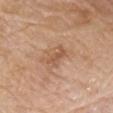| key | value |
|---|---|
| notes | no biopsy performed (imaged during a skin exam) |
| size | about 3.5 mm |
| patient | male, aged 78–82 |
| anatomic site | the chest |
| lighting | white-light |
| image source | ~15 mm crop, total-body skin-cancer survey |
| image-analysis metrics | an area of roughly 3.5 mm², a shape eccentricity near 0.9, and a shape-asymmetry score of about 0.3 (0 = symmetric); a border-irregularity rating of about 4.5/10 and a peripheral color-asymmetry measure near 0 |